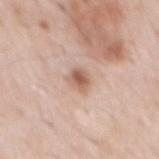  biopsy_status: not biopsied; imaged during a skin examination
  patient:
    sex: male
    age_approx: 60
  automated_metrics:
    area_mm2_approx: 4.5
    eccentricity: 0.65
    shape_asymmetry: 0.25
    nevus_likeness_0_100: 85
    lesion_detection_confidence_0_100: 100
  lighting: white-light
  site: mid back
  lesion_size:
    long_diameter_mm_approx: 3.0
  image:
    source: total-body photography crop
    field_of_view_mm: 15